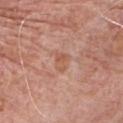{
  "biopsy_status": "not biopsied; imaged during a skin examination",
  "automated_metrics": {
    "cielab_L": 57,
    "cielab_a": 23,
    "cielab_b": 30,
    "vs_skin_darker_L": 6.0,
    "vs_skin_contrast_norm": 5.0,
    "nevus_likeness_0_100": 0,
    "lesion_detection_confidence_0_100": 100
  },
  "patient": {
    "sex": "male",
    "age_approx": 80
  },
  "lesion_size": {
    "long_diameter_mm_approx": 3.0
  },
  "site": "chest",
  "lighting": "white-light",
  "image": {
    "source": "total-body photography crop",
    "field_of_view_mm": 15
  }
}